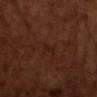Captured during whole-body skin photography for melanoma surveillance; the lesion was not biopsied. The tile uses cross-polarized illumination. On the chest. The recorded lesion diameter is about 2.5 mm. A male subject aged 63–67. A close-up tile cropped from a whole-body skin photograph, about 15 mm across. Automated tile analysis of the lesion measured a footprint of about 2.5 mm² and two-axis asymmetry of about 0.3. And it measured an automated nevus-likeness rating near 5 out of 100 and a detector confidence of about 90 out of 100 that the crop contains a lesion.